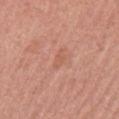Part of a total-body skin-imaging series; this lesion was reviewed on a skin check and was not flagged for biopsy. From the left upper arm. A 15 mm close-up extracted from a 3D total-body photography capture. Imaged with white-light lighting. A female patient, roughly 55 years of age. The recorded lesion diameter is about 2.5 mm. An algorithmic analysis of the crop reported a normalized lesion–skin contrast near 4.5. The analysis additionally found a border-irregularity index near 3.5/10, a color-variation rating of about 0/10, and peripheral color asymmetry of about 0. The analysis additionally found a classifier nevus-likeness of about 0/100 and a lesion-detection confidence of about 100/100.